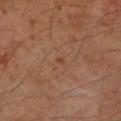Q: Was this lesion biopsied?
A: no biopsy performed (imaged during a skin exam)
Q: What are the patient's age and sex?
A: female, in their 60s
Q: What did automated image analysis measure?
A: a footprint of about 1 mm², a shape eccentricity near 0.6, and a symmetry-axis asymmetry near 0.5
Q: What is the anatomic site?
A: the left forearm
Q: Illumination type?
A: cross-polarized
Q: What is the imaging modality?
A: ~15 mm tile from a whole-body skin photo